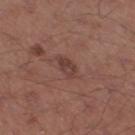workup = no biopsy performed (imaged during a skin exam)
tile lighting = white-light illumination
lesion diameter = ≈3 mm
imaging modality = 15 mm crop, total-body photography
patient = male, in their mid- to late 50s
body site = the right thigh